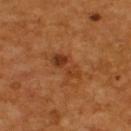Q: Was this lesion biopsied?
A: total-body-photography surveillance lesion; no biopsy
Q: Patient demographics?
A: male, roughly 55 years of age
Q: Lesion location?
A: the back
Q: What is the imaging modality?
A: ~15 mm crop, total-body skin-cancer survey